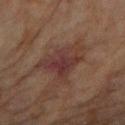workup=total-body-photography surveillance lesion; no biopsy | site=the right forearm | illumination=cross-polarized | imaging modality=15 mm crop, total-body photography | automated metrics=a lesion color around L≈29 a*≈18 b*≈19 in CIELAB and a normalized lesion–skin contrast near 7.5; border irregularity of about 5.5 on a 0–10 scale, a color-variation rating of about 4/10, and a peripheral color-asymmetry measure near 1 | patient=female, in their 80s | size=~5.5 mm (longest diameter).